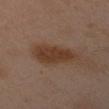  lesion_size:
    long_diameter_mm_approx: 5.5
  site: left forearm
  image:
    source: total-body photography crop
    field_of_view_mm: 15
  patient:
    sex: female
    age_approx: 40
  automated_metrics:
    cielab_L: 38
    cielab_a: 18
    cielab_b: 28
    vs_skin_darker_L: 9.0
    vs_skin_contrast_norm: 9.0
    border_irregularity_0_10: 2.5
    color_variation_0_10: 2.0
    peripheral_color_asymmetry: 0.5
    nevus_likeness_0_100: 100
  lighting: cross-polarized